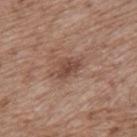{
  "site": "upper back",
  "lesion_size": {
    "long_diameter_mm_approx": 3.0
  },
  "image": {
    "source": "total-body photography crop",
    "field_of_view_mm": 15
  },
  "patient": {
    "sex": "male",
    "age_approx": 70
  }
}